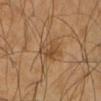The lesion was photographed on a routine skin check and not biopsied; there is no pathology result.
Located on the right arm.
The lesion-visualizer software estimated a lesion area of about 7 mm², an eccentricity of roughly 0.85, and a shape-asymmetry score of about 0.25 (0 = symmetric). It also reported a lesion–skin lightness drop of about 7 and a normalized lesion–skin contrast near 6. The software also gave a border-irregularity rating of about 3.5/10 and peripheral color asymmetry of about 2.
The subject is a male approximately 60 years of age.
The tile uses cross-polarized illumination.
A 15 mm close-up tile from a total-body photography series done for melanoma screening.